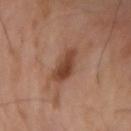Captured during whole-body skin photography for melanoma surveillance; the lesion was not biopsied. The lesion's longest dimension is about 4 mm. Captured under cross-polarized illumination. The total-body-photography lesion software estimated a mean CIELAB color near L≈42 a*≈21 b*≈28 and a lesion–skin lightness drop of about 11. And it measured a nevus-likeness score of about 70/100 and a detector confidence of about 100 out of 100 that the crop contains a lesion. Cropped from a whole-body photographic skin survey; the tile spans about 15 mm. A male subject about 65 years old.